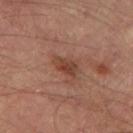No biopsy was performed on this lesion — it was imaged during a full skin examination and was not determined to be concerning.
A male subject in their mid-60s.
Longest diameter approximately 3 mm.
A lesion tile, about 15 mm wide, cut from a 3D total-body photograph.
On the left thigh.